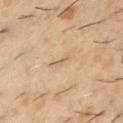Captured during whole-body skin photography for melanoma surveillance; the lesion was not biopsied. Cropped from a total-body skin-imaging series; the visible field is about 15 mm. A male patient, aged approximately 55. Approximately 2.5 mm at its widest. An algorithmic analysis of the crop reported a shape-asymmetry score of about 0.35 (0 = symmetric). It also reported a lesion color around L≈61 a*≈15 b*≈33 in CIELAB, roughly 8 lightness units darker than nearby skin, and a normalized border contrast of about 5. It also reported a border-irregularity index near 3.5/10, a within-lesion color-variation index near 0/10, and a peripheral color-asymmetry measure near 0. The tile uses cross-polarized illumination. The lesion is located on the chest.